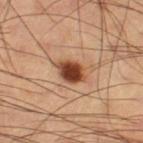* biopsy status · catalogued during a skin exam; not biopsied
* lesion diameter · ~3.5 mm (longest diameter)
* subject · male, aged around 60
* imaging modality · 15 mm crop, total-body photography
* site · the right thigh
* illumination · cross-polarized illumination